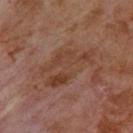follow-up = imaged on a skin check; not biopsied | TBP lesion metrics = a nevus-likeness score of about 0/100 and a lesion-detection confidence of about 100/100 | lighting = cross-polarized | location = the upper back | patient = male, about 70 years old | lesion diameter = about 6.5 mm | image = ~15 mm tile from a whole-body skin photo.